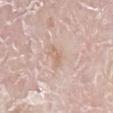Q: Was a biopsy performed?
A: catalogued during a skin exam; not biopsied
Q: What kind of image is this?
A: 15 mm crop, total-body photography
Q: What are the patient's age and sex?
A: male, aged 78–82
Q: Lesion location?
A: the right lower leg
Q: What is the lesion's diameter?
A: ≈2.5 mm
Q: Illumination type?
A: white-light